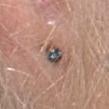Q: Is there a histopathology result?
A: imaged on a skin check; not biopsied
Q: What is the lesion's diameter?
A: ≈3.5 mm
Q: Where on the body is the lesion?
A: the head or neck
Q: What are the patient's age and sex?
A: female, approximately 45 years of age
Q: What did automated image analysis measure?
A: a mean CIELAB color near L≈48 a*≈11 b*≈18, about 14 CIELAB-L* units darker than the surrounding skin, and a normalized border contrast of about 11; border irregularity of about 2 on a 0–10 scale
Q: How was the tile lit?
A: white-light
Q: What is the imaging modality?
A: total-body-photography crop, ~15 mm field of view